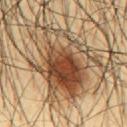Clinical impression: No biopsy was performed on this lesion — it was imaged during a full skin examination and was not determined to be concerning. Clinical summary: Located on the mid back. A 15 mm close-up extracted from a 3D total-body photography capture. A male patient, aged approximately 45. Approximately 9 mm at its widest.